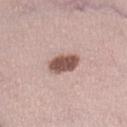biopsy status: no biopsy performed (imaged during a skin exam)
image: 15 mm crop, total-body photography
lighting: white-light illumination
location: the right lower leg
patient: female, aged around 35
size: ≈3.5 mm